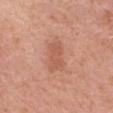Q: Is there a histopathology result?
A: imaged on a skin check; not biopsied
Q: What is the lesion's diameter?
A: ~3.5 mm (longest diameter)
Q: How was this image acquired?
A: total-body-photography crop, ~15 mm field of view
Q: Automated lesion metrics?
A: a lesion area of about 7 mm², an outline eccentricity of about 0.85 (0 = round, 1 = elongated), and a shape-asymmetry score of about 0.3 (0 = symmetric); a lesion–skin lightness drop of about 8 and a normalized lesion–skin contrast near 5.5
Q: Lesion location?
A: the left forearm
Q: How was the tile lit?
A: white-light
Q: Patient demographics?
A: female, in their 40s